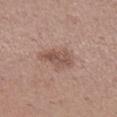Clinical impression: No biopsy was performed on this lesion — it was imaged during a full skin examination and was not determined to be concerning. Background: Measured at roughly 4 mm in maximum diameter. Cropped from a whole-body photographic skin survey; the tile spans about 15 mm. Imaged with white-light lighting. Automated tile analysis of the lesion measured an area of roughly 7 mm², an outline eccentricity of about 0.85 (0 = round, 1 = elongated), and two-axis asymmetry of about 0.3. And it measured an average lesion color of about L≈52 a*≈19 b*≈25 (CIELAB), a lesion–skin lightness drop of about 9, and a normalized lesion–skin contrast near 7. The analysis additionally found a border-irregularity rating of about 3.5/10, internal color variation of about 3.5 on a 0–10 scale, and a peripheral color-asymmetry measure near 1. From the right lower leg. The patient is a female about 30 years old.